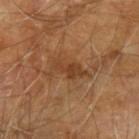A close-up tile cropped from a whole-body skin photograph, about 15 mm across. The lesion is on the left upper arm. This is a cross-polarized tile. The lesion's longest dimension is about 4.5 mm. A male patient, roughly 70 years of age.